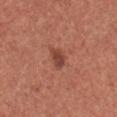No biopsy was performed on this lesion — it was imaged during a full skin examination and was not determined to be concerning.
Measured at roughly 2.5 mm in maximum diameter.
This image is a 15 mm lesion crop taken from a total-body photograph.
The total-body-photography lesion software estimated an average lesion color of about L≈44 a*≈26 b*≈28 (CIELAB), about 10 CIELAB-L* units darker than the surrounding skin, and a normalized border contrast of about 8. And it measured lesion-presence confidence of about 100/100.
The lesion is located on the upper back.
Imaged with white-light lighting.
The subject is a female aged approximately 35.